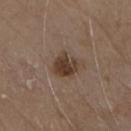This lesion was catalogued during total-body skin photography and was not selected for biopsy. The recorded lesion diameter is about 3 mm. This is a white-light tile. Automated image analysis of the tile measured a lesion area of about 7 mm², an outline eccentricity of about 0.5 (0 = round, 1 = elongated), and a shape-asymmetry score of about 0.3 (0 = symmetric). It also reported a mean CIELAB color near L≈38 a*≈15 b*≈25. The lesion is on the chest. A 15 mm crop from a total-body photograph taken for skin-cancer surveillance. A male patient in their 80s.